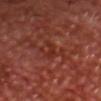Q: Was a biopsy performed?
A: imaged on a skin check; not biopsied
Q: Automated lesion metrics?
A: a lesion area of about 2.5 mm², an eccentricity of roughly 0.95, and two-axis asymmetry of about 0.55; a lesion color around L≈30 a*≈28 b*≈28 in CIELAB and a normalized border contrast of about 5.5; a within-lesion color-variation index near 0/10 and a peripheral color-asymmetry measure near 0; an automated nevus-likeness rating near 0 out of 100 and a detector confidence of about 65 out of 100 that the crop contains a lesion
Q: What kind of image is this?
A: ~15 mm crop, total-body skin-cancer survey
Q: What lighting was used for the tile?
A: cross-polarized illumination
Q: What are the patient's age and sex?
A: male, about 65 years old
Q: How large is the lesion?
A: about 3 mm